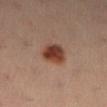This lesion was catalogued during total-body skin photography and was not selected for biopsy. A female patient, aged around 35. The lesion-visualizer software estimated roughly 13 lightness units darker than nearby skin and a normalized lesion–skin contrast near 11. The lesion's longest dimension is about 3.5 mm. This image is a 15 mm lesion crop taken from a total-body photograph. This is a cross-polarized tile. The lesion is located on the left lower leg.